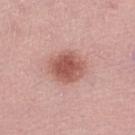The lesion was photographed on a routine skin check and not biopsied; there is no pathology result. A 15 mm crop from a total-body photograph taken for skin-cancer surveillance. A female patient, aged approximately 60. On the leg. Automated tile analysis of the lesion measured a lesion area of about 12 mm², a shape eccentricity near 0.5, and a shape-asymmetry score of about 0.1 (0 = symmetric). It also reported a lesion color around L≈55 a*≈26 b*≈26 in CIELAB, about 13 CIELAB-L* units darker than the surrounding skin, and a lesion-to-skin contrast of about 8.5 (normalized; higher = more distinct). It also reported a border-irregularity rating of about 1/10, a within-lesion color-variation index near 3.5/10, and a peripheral color-asymmetry measure near 1. Captured under white-light illumination. The lesion's longest dimension is about 4 mm.